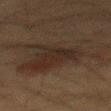diameter: about 9.5 mm
image: total-body-photography crop, ~15 mm field of view
tile lighting: cross-polarized
body site: the back
patient: male, roughly 35 years of age